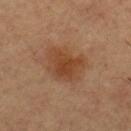Notes:
- follow-up — total-body-photography surveillance lesion; no biopsy
- imaging modality — total-body-photography crop, ~15 mm field of view
- tile lighting — cross-polarized
- lesion size — about 4.5 mm
- subject — male, aged 63–67
- location — the upper back